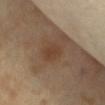Captured during whole-body skin photography for melanoma surveillance; the lesion was not biopsied. On the abdomen. A female patient aged 53–57. An algorithmic analysis of the crop reported an outline eccentricity of about 0.65 (0 = round, 1 = elongated) and a shape-asymmetry score of about 0.25 (0 = symmetric). The analysis additionally found a mean CIELAB color near L≈41 a*≈18 b*≈29 and a lesion–skin lightness drop of about 6. The analysis additionally found a border-irregularity rating of about 2/10, a color-variation rating of about 1/10, and radial color variation of about 0.5. A close-up tile cropped from a whole-body skin photograph, about 15 mm across. The recorded lesion diameter is about 2.5 mm. Captured under cross-polarized illumination.